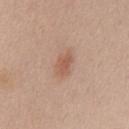image source: total-body-photography crop, ~15 mm field of view
site: the chest
diameter: about 3 mm
subject: female, aged around 45
automated metrics: an area of roughly 4.5 mm² and a shape eccentricity near 0.8; border irregularity of about 2.5 on a 0–10 scale, a within-lesion color-variation index near 1.5/10, and peripheral color asymmetry of about 0.5